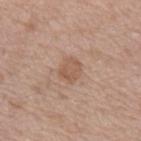Impression: Part of a total-body skin-imaging series; this lesion was reviewed on a skin check and was not flagged for biopsy. Image and clinical context: An algorithmic analysis of the crop reported a lesion–skin lightness drop of about 8 and a normalized border contrast of about 5.5. The analysis additionally found a border-irregularity rating of about 2/10 and a color-variation rating of about 2/10. And it measured an automated nevus-likeness rating near 5 out of 100 and a detector confidence of about 100 out of 100 that the crop contains a lesion. A male subject, aged 43–47. The lesion's longest dimension is about 2.5 mm. A region of skin cropped from a whole-body photographic capture, roughly 15 mm wide. The lesion is on the arm.